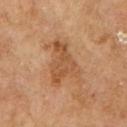Part of a total-body skin-imaging series; this lesion was reviewed on a skin check and was not flagged for biopsy.
Automated tile analysis of the lesion measured an outline eccentricity of about 0.75 (0 = round, 1 = elongated) and a shape-asymmetry score of about 0.55 (0 = symmetric). It also reported a lesion–skin lightness drop of about 9 and a normalized border contrast of about 6.5. It also reported border irregularity of about 6.5 on a 0–10 scale, a color-variation rating of about 3/10, and peripheral color asymmetry of about 1. It also reported a classifier nevus-likeness of about 0/100 and lesion-presence confidence of about 100/100.
Imaged with cross-polarized lighting.
The subject is a male aged around 70.
A lesion tile, about 15 mm wide, cut from a 3D total-body photograph.
On the right upper arm.